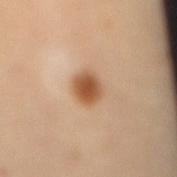An algorithmic analysis of the crop reported a lesion-detection confidence of about 100/100.
The lesion is located on the mid back.
A female subject in their 60s.
A roughly 15 mm field-of-view crop from a total-body skin photograph.
The lesion's longest dimension is about 3.5 mm.
Imaged with cross-polarized lighting.